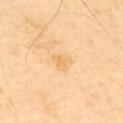Q: Is there a histopathology result?
A: no biopsy performed (imaged during a skin exam)
Q: What is the lesion's diameter?
A: about 2.5 mm
Q: Lesion location?
A: the upper back
Q: Patient demographics?
A: male, aged around 65
Q: Illumination type?
A: cross-polarized illumination
Q: What is the imaging modality?
A: 15 mm crop, total-body photography